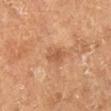notes: total-body-photography surveillance lesion; no biopsy
acquisition: ~15 mm crop, total-body skin-cancer survey
lighting: cross-polarized
anatomic site: the leg
patient: female, aged 63 to 67
lesion size: about 3 mm